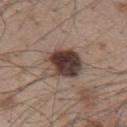Recorded during total-body skin imaging; not selected for excision or biopsy.
The patient is a male in their 50s.
This image is a 15 mm lesion crop taken from a total-body photograph.
The lesion is on the upper back.